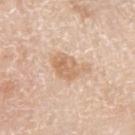No biopsy was performed on this lesion — it was imaged during a full skin examination and was not determined to be concerning. Located on the left upper arm. A male patient, aged around 80. A region of skin cropped from a whole-body photographic capture, roughly 15 mm wide. The lesion-visualizer software estimated an automated nevus-likeness rating near 30 out of 100 and a detector confidence of about 100 out of 100 that the crop contains a lesion.